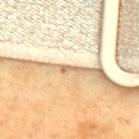Case summary:
– image source · ~15 mm tile from a whole-body skin photo
– patient · roughly 70 years of age
– tile lighting · cross-polarized
– lesion size · ~2.5 mm (longest diameter)
– anatomic site · the back